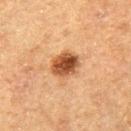The lesion was photographed on a routine skin check and not biopsied; there is no pathology result. The lesion-visualizer software estimated a shape eccentricity near 0.45 and a shape-asymmetry score of about 0.1 (0 = symmetric). And it measured border irregularity of about 1 on a 0–10 scale and internal color variation of about 6 on a 0–10 scale. A 15 mm close-up tile from a total-body photography series done for melanoma screening. A male patient about 75 years old. About 3.5 mm across. Imaged with cross-polarized lighting. On the right thigh.